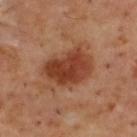biopsy status=no biopsy performed (imaged during a skin exam) | anatomic site=the upper back | patient=male, about 55 years old | image=~15 mm crop, total-body skin-cancer survey.